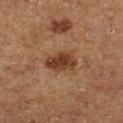The lesion was photographed on a routine skin check and not biopsied; there is no pathology result.